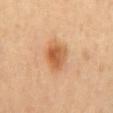Case summary:
- notes · catalogued during a skin exam; not biopsied
- image-analysis metrics · an area of roughly 8.5 mm², an eccentricity of roughly 0.65, and a shape-asymmetry score of about 0.2 (0 = symmetric); an automated nevus-likeness rating near 100 out of 100 and a detector confidence of about 100 out of 100 that the crop contains a lesion
- patient · female, about 65 years old
- location · the mid back
- diameter · ≈3.5 mm
- imaging modality · ~15 mm crop, total-body skin-cancer survey